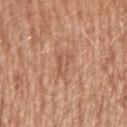| field | value |
|---|---|
| notes | total-body-photography surveillance lesion; no biopsy |
| image-analysis metrics | a border-irregularity rating of about 5/10 and a color-variation rating of about 2.5/10 |
| imaging modality | 15 mm crop, total-body photography |
| anatomic site | the right upper arm |
| diameter | about 3.5 mm |
| patient | female, aged 38 to 42 |